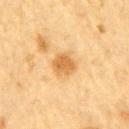biopsy status — no biopsy performed (imaged during a skin exam)
diameter — ≈3 mm
site — the left upper arm
tile lighting — cross-polarized illumination
acquisition — ~15 mm tile from a whole-body skin photo
subject — male, aged approximately 85 or older